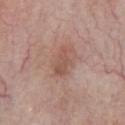patient = male, approximately 60 years of age
location = the front of the torso
diameter = ~3.5 mm (longest diameter)
lighting = white-light
image = ~15 mm tile from a whole-body skin photo
automated lesion analysis = a footprint of about 5 mm², a shape eccentricity near 0.9, and a symmetry-axis asymmetry near 0.35; border irregularity of about 3.5 on a 0–10 scale; a nevus-likeness score of about 5/100